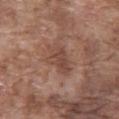{
  "biopsy_status": "not biopsied; imaged during a skin examination",
  "image": {
    "source": "total-body photography crop",
    "field_of_view_mm": 15
  },
  "lesion_size": {
    "long_diameter_mm_approx": 4.0
  },
  "site": "front of the torso",
  "automated_metrics": {
    "shape_asymmetry": 0.3,
    "vs_skin_darker_L": 8.0,
    "vs_skin_contrast_norm": 6.0,
    "nevus_likeness_0_100": 0,
    "lesion_detection_confidence_0_100": 100
  },
  "lighting": "white-light",
  "patient": {
    "sex": "male",
    "age_approx": 75
  }
}